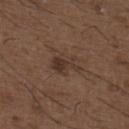<tbp_lesion>
  <biopsy_status>not biopsied; imaged during a skin examination</biopsy_status>
  <lighting>white-light</lighting>
  <automated_metrics>
    <nevus_likeness_0_100>45</nevus_likeness_0_100>
    <lesion_detection_confidence_0_100>100</lesion_detection_confidence_0_100>
  </automated_metrics>
  <patient>
    <sex>male</sex>
    <age_approx>50</age_approx>
  </patient>
  <lesion_size>
    <long_diameter_mm_approx>4.0</long_diameter_mm_approx>
  </lesion_size>
  <site>back</site>
  <image>
    <source>total-body photography crop</source>
    <field_of_view_mm>15</field_of_view_mm>
  </image>
</tbp_lesion>